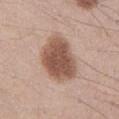workup: total-body-photography surveillance lesion; no biopsy | automated lesion analysis: a lesion area of about 21 mm², a shape eccentricity near 0.75, and a shape-asymmetry score of about 0.2 (0 = symmetric); radial color variation of about 1; a nevus-likeness score of about 95/100 and a lesion-detection confidence of about 100/100 | imaging modality: total-body-photography crop, ~15 mm field of view | subject: male, aged 33–37 | location: the mid back | illumination: white-light illumination | diameter: ~6.5 mm (longest diameter).